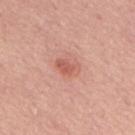<case>
  <biopsy_status>not biopsied; imaged during a skin examination</biopsy_status>
  <lighting>white-light</lighting>
  <automated_metrics>
    <area_mm2_approx>4.5</area_mm2_approx>
    <shape_asymmetry>0.25</shape_asymmetry>
    <border_irregularity_0_10>2.5</border_irregularity_0_10>
    <color_variation_0_10>2.5</color_variation_0_10>
    <peripheral_color_asymmetry>1.0</peripheral_color_asymmetry>
  </automated_metrics>
  <patient>
    <sex>male</sex>
    <age_approx>75</age_approx>
  </patient>
  <lesion_size>
    <long_diameter_mm_approx>3.0</long_diameter_mm_approx>
  </lesion_size>
  <image>
    <source>total-body photography crop</source>
    <field_of_view_mm>15</field_of_view_mm>
  </image>
  <site>mid back</site>
</case>